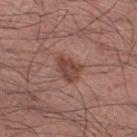Imaged during a routine full-body skin examination; the lesion was not biopsied and no histopathology is available.